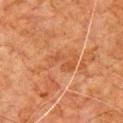Assessment: No biopsy was performed on this lesion — it was imaged during a full skin examination and was not determined to be concerning. Context: This is a cross-polarized tile. The lesion is on the chest. A male subject roughly 80 years of age. A 15 mm close-up tile from a total-body photography series done for melanoma screening.